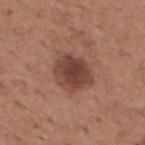location: the back | patient: male, aged 63 to 67 | acquisition: 15 mm crop, total-body photography.